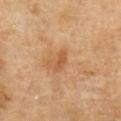Findings:
– notes — imaged on a skin check; not biopsied
– lighting — cross-polarized
– site — the left forearm
– image — 15 mm crop, total-body photography
– diameter — ≈2.5 mm
– subject — female, aged 63–67